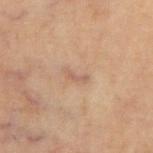workup = catalogued during a skin exam; not biopsied
diameter = ≈2.5 mm
imaging modality = 15 mm crop, total-body photography
site = the chest
automated metrics = a footprint of about 2 mm², a shape eccentricity near 0.95, and two-axis asymmetry of about 0.45; a mean CIELAB color near L≈44 a*≈15 b*≈22 and a lesion–skin lightness drop of about 6; a border-irregularity rating of about 5/10, internal color variation of about 0 on a 0–10 scale, and radial color variation of about 0
lighting = cross-polarized illumination
patient = male, about 70 years old